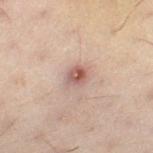The lesion was tiled from a total-body skin photograph and was not biopsied. The patient is a female in their 30s. The lesion is located on the left leg. A 15 mm crop from a total-body photograph taken for skin-cancer surveillance. Imaged with cross-polarized lighting. The recorded lesion diameter is about 3 mm.